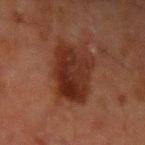Clinical impression: This lesion was catalogued during total-body skin photography and was not selected for biopsy. Context: The lesion is located on the left upper arm. A male subject, approximately 60 years of age. A 15 mm crop from a total-body photograph taken for skin-cancer surveillance.